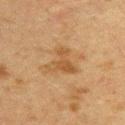Recorded during total-body skin imaging; not selected for excision or biopsy.
The lesion's longest dimension is about 4.5 mm.
This image is a 15 mm lesion crop taken from a total-body photograph.
From the upper back.
Automated image analysis of the tile measured a footprint of about 8.5 mm² and a shape eccentricity near 0.45. And it measured border irregularity of about 7 on a 0–10 scale, internal color variation of about 3 on a 0–10 scale, and radial color variation of about 1.
The patient is a female about 55 years old.
Imaged with cross-polarized lighting.